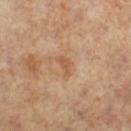  biopsy_status: not biopsied; imaged during a skin examination
  lesion_size:
    long_diameter_mm_approx: 2.5
  automated_metrics:
    area_mm2_approx: 2.5
    eccentricity: 0.85
    shape_asymmetry: 0.45
    cielab_L: 53
    cielab_a: 20
    cielab_b: 34
    vs_skin_contrast_norm: 6.0
  site: leg
  patient:
    sex: female
    age_approx: 65
  image:
    source: total-body photography crop
    field_of_view_mm: 15
  lighting: cross-polarized